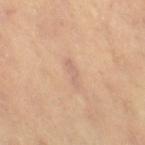– TBP lesion metrics · a border-irregularity index near 6.5/10; a nevus-likeness score of about 0/100 and a lesion-detection confidence of about 90/100
– tile lighting · cross-polarized illumination
– patient · female, aged 68–72
– imaging modality · ~15 mm crop, total-body skin-cancer survey
– lesion diameter · ≈3 mm
– body site · the right thigh